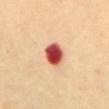Image and clinical context: A female patient, about 60 years old. A 15 mm close-up tile from a total-body photography series done for melanoma screening. The lesion is on the abdomen.